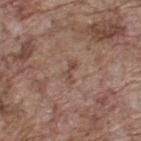| field | value |
|---|---|
| follow-up | catalogued during a skin exam; not biopsied |
| imaging modality | 15 mm crop, total-body photography |
| lighting | white-light |
| TBP lesion metrics | a footprint of about 2.5 mm²; a normalized border contrast of about 6; a border-irregularity rating of about 5/10 and radial color variation of about 0 |
| site | the upper back |
| lesion size | ~2.5 mm (longest diameter) |
| patient | male, aged around 70 |